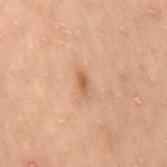The lesion was photographed on a routine skin check and not biopsied; there is no pathology result. The patient is a female about 55 years old. About 2.5 mm across. From the left leg. A roughly 15 mm field-of-view crop from a total-body skin photograph. Captured under cross-polarized illumination.